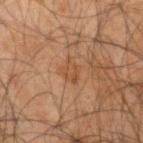Findings:
- biopsy status: catalogued during a skin exam; not biopsied
- acquisition: ~15 mm tile from a whole-body skin photo
- subject: male, aged approximately 65
- location: the right upper arm
- lesion size: ~2.5 mm (longest diameter)
- automated metrics: a border-irregularity index near 3/10 and a color-variation rating of about 2/10